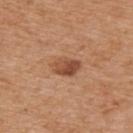Assessment: Part of a total-body skin-imaging series; this lesion was reviewed on a skin check and was not flagged for biopsy. Clinical summary: The lesion's longest dimension is about 3.5 mm. Located on the upper back. A roughly 15 mm field-of-view crop from a total-body skin photograph. Imaged with white-light lighting. A male patient aged approximately 65.